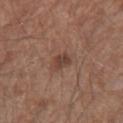Q: Was this lesion biopsied?
A: catalogued during a skin exam; not biopsied
Q: What is the imaging modality?
A: total-body-photography crop, ~15 mm field of view
Q: Who is the patient?
A: male, roughly 55 years of age
Q: Automated lesion metrics?
A: a nevus-likeness score of about 40/100 and lesion-presence confidence of about 100/100
Q: Where on the body is the lesion?
A: the right upper arm